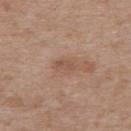Clinical summary:
Located on the upper back. This is a white-light tile. A close-up tile cropped from a whole-body skin photograph, about 15 mm across. A male subject, about 70 years old.